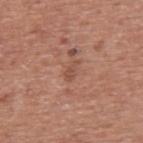Assessment:
Captured during whole-body skin photography for melanoma surveillance; the lesion was not biopsied.
Context:
The lesion's longest dimension is about 2.5 mm. Located on the back. An algorithmic analysis of the crop reported a border-irregularity rating of about 4.5/10 and a peripheral color-asymmetry measure near 0. A close-up tile cropped from a whole-body skin photograph, about 15 mm across. A male subject roughly 75 years of age. Imaged with white-light lighting.